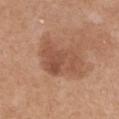Q: Is there a histopathology result?
A: no biopsy performed (imaged during a skin exam)
Q: What is the lesion's diameter?
A: ≈5.5 mm
Q: How was the tile lit?
A: white-light illumination
Q: What are the patient's age and sex?
A: female, aged 53–57
Q: What kind of image is this?
A: ~15 mm tile from a whole-body skin photo
Q: Where on the body is the lesion?
A: the chest